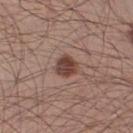Findings:
* site · the right lower leg
* lesion size · ≈2.5 mm
* TBP lesion metrics · an area of roughly 5.5 mm² and a shape eccentricity near 0.4; a mean CIELAB color near L≈42 a*≈20 b*≈23
* subject · male, roughly 30 years of age
* acquisition · total-body-photography crop, ~15 mm field of view
* tile lighting · white-light